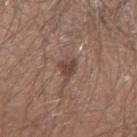Q: Was this lesion biopsied?
A: imaged on a skin check; not biopsied
Q: Who is the patient?
A: male, in their 30s
Q: Illumination type?
A: white-light illumination
Q: How was this image acquired?
A: ~15 mm crop, total-body skin-cancer survey
Q: Where on the body is the lesion?
A: the left forearm